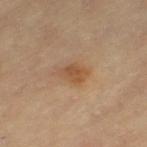Acquisition and patient details:
From the left thigh. Imaged with cross-polarized lighting. A female patient aged approximately 70. Automated image analysis of the tile measured a lesion color around L≈53 a*≈19 b*≈35 in CIELAB, about 8 CIELAB-L* units darker than the surrounding skin, and a normalized border contrast of about 6.5. And it measured a border-irregularity index near 2/10, a within-lesion color-variation index near 3.5/10, and peripheral color asymmetry of about 1. A 15 mm close-up extracted from a 3D total-body photography capture. The recorded lesion diameter is about 3 mm.